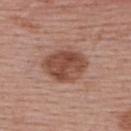• follow-up — imaged on a skin check; not biopsied